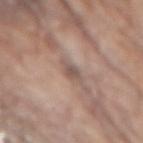• biopsy status: no biopsy performed (imaged during a skin exam)
• illumination: white-light illumination
• patient: male, approximately 80 years of age
• anatomic site: the mid back
• lesion diameter: ~2.5 mm (longest diameter)
• image source: ~15 mm tile from a whole-body skin photo